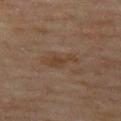No biopsy was performed on this lesion — it was imaged during a full skin examination and was not determined to be concerning.
The lesion is located on the left thigh.
A female patient, aged 58–62.
A region of skin cropped from a whole-body photographic capture, roughly 15 mm wide.
Imaged with cross-polarized lighting.
Approximately 4 mm at its widest.
The lesion-visualizer software estimated an eccentricity of roughly 0.95 and a shape-asymmetry score of about 0.4 (0 = symmetric). The software also gave a border-irregularity index near 5/10, internal color variation of about 1 on a 0–10 scale, and peripheral color asymmetry of about 0.5. It also reported an automated nevus-likeness rating near 0 out of 100.